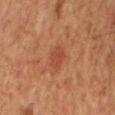tile lighting = cross-polarized
image source = ~15 mm tile from a whole-body skin photo
location = the chest
subject = male, aged approximately 60
image-analysis metrics = a lesion area of about 4 mm², an eccentricity of roughly 0.75, and two-axis asymmetry of about 0.25; radial color variation of about 0.5; a classifier nevus-likeness of about 45/100